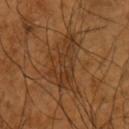Impression:
Part of a total-body skin-imaging series; this lesion was reviewed on a skin check and was not flagged for biopsy.
Clinical summary:
The recorded lesion diameter is about 7 mm. The subject is a male aged approximately 65. A lesion tile, about 15 mm wide, cut from a 3D total-body photograph. The lesion is located on the left upper arm. Imaged with cross-polarized lighting.